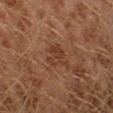Recorded during total-body skin imaging; not selected for excision or biopsy. Captured under cross-polarized illumination. Measured at roughly 3.5 mm in maximum diameter. A close-up tile cropped from a whole-body skin photograph, about 15 mm across. The patient is a male aged around 30. The lesion is on the left lower leg. The total-body-photography lesion software estimated a footprint of about 6 mm², an eccentricity of roughly 0.6, and two-axis asymmetry of about 0.4. And it measured an average lesion color of about L≈37 a*≈22 b*≈30 (CIELAB), roughly 7 lightness units darker than nearby skin, and a lesion-to-skin contrast of about 6 (normalized; higher = more distinct). The analysis additionally found a nevus-likeness score of about 0/100.